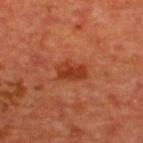Case summary:
* notes: no biopsy performed (imaged during a skin exam)
* image: ~15 mm crop, total-body skin-cancer survey
* illumination: cross-polarized
* subject: male, in their mid- to late 60s
* size: ≈3.5 mm
* location: the back